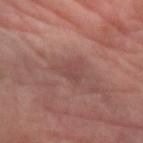follow-up = imaged on a skin check; not biopsied, anatomic site = the left forearm, image-analysis metrics = a normalized lesion–skin contrast near 5; a classifier nevus-likeness of about 0/100, size = ≈4 mm, image source = ~15 mm tile from a whole-body skin photo, illumination = cross-polarized illumination.